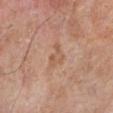biopsy_status: not biopsied; imaged during a skin examination
lesion_size:
  long_diameter_mm_approx: 3.0
patient:
  sex: male
  age_approx: 80
site: left lower leg
lighting: white-light
image:
  source: total-body photography crop
  field_of_view_mm: 15